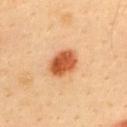This lesion was catalogued during total-body skin photography and was not selected for biopsy. Cropped from a total-body skin-imaging series; the visible field is about 15 mm. The lesion-visualizer software estimated an outline eccentricity of about 0.7 (0 = round, 1 = elongated) and a shape-asymmetry score of about 0.15 (0 = symmetric). The subject is a male about 40 years old. Captured under cross-polarized illumination. The lesion is on the upper back. Approximately 4 mm at its widest.